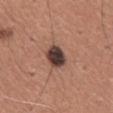Q: What is the anatomic site?
A: the mid back
Q: Patient demographics?
A: male, aged 43–47
Q: Automated lesion metrics?
A: a lesion color around L≈40 a*≈17 b*≈21 in CIELAB and a normalized lesion–skin contrast near 14; border irregularity of about 2 on a 0–10 scale, internal color variation of about 6 on a 0–10 scale, and a peripheral color-asymmetry measure near 1.5; a classifier nevus-likeness of about 45/100 and lesion-presence confidence of about 100/100
Q: How was the tile lit?
A: white-light
Q: What is the imaging modality?
A: total-body-photography crop, ~15 mm field of view
Q: What is the lesion's diameter?
A: ≈3 mm